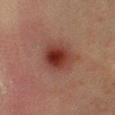imaging modality: ~15 mm crop, total-body skin-cancer survey | lighting: cross-polarized illumination | size: about 4 mm | site: the right lower leg | subject: male, roughly 60 years of age.